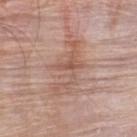Q: Was this lesion biopsied?
A: no biopsy performed (imaged during a skin exam)
Q: What lighting was used for the tile?
A: white-light
Q: Lesion size?
A: about 7.5 mm
Q: Where on the body is the lesion?
A: the upper back
Q: How was this image acquired?
A: total-body-photography crop, ~15 mm field of view
Q: Patient demographics?
A: male, aged approximately 50